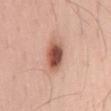{"biopsy_status": "not biopsied; imaged during a skin examination", "lighting": "white-light", "image": {"source": "total-body photography crop", "field_of_view_mm": 15}, "site": "lower back", "patient": {"sex": "male", "age_approx": 35}}